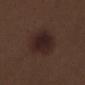{"biopsy_status": "not biopsied; imaged during a skin examination", "patient": {"sex": "male", "age_approx": 70}, "image": {"source": "total-body photography crop", "field_of_view_mm": 15}, "site": "left thigh", "automated_metrics": {"color_variation_0_10": 3.0, "nevus_likeness_0_100": 85, "lesion_detection_confidence_0_100": 100}, "lighting": "white-light"}